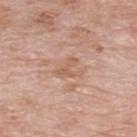  biopsy_status: not biopsied; imaged during a skin examination
  lesion_size:
    long_diameter_mm_approx: 3.0
  site: upper back
  patient:
    sex: male
    age_approx: 60
  image:
    source: total-body photography crop
    field_of_view_mm: 15
  lighting: white-light
  automated_metrics:
    area_mm2_approx: 5.5
    eccentricity: 0.45
    cielab_L: 60
    cielab_a: 20
    cielab_b: 30
    border_irregularity_0_10: 6.0
    color_variation_0_10: 3.0
    peripheral_color_asymmetry: 1.0
    nevus_likeness_0_100: 0
    lesion_detection_confidence_0_100: 100